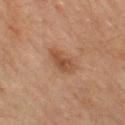Imaged during a routine full-body skin examination; the lesion was not biopsied and no histopathology is available. A female subject, about 55 years old. Cropped from a whole-body photographic skin survey; the tile spans about 15 mm. On the left thigh.